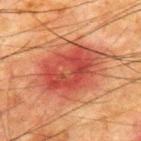Impression: No biopsy was performed on this lesion — it was imaged during a full skin examination and was not determined to be concerning. Acquisition and patient details: A roughly 15 mm field-of-view crop from a total-body skin photograph. A male patient, aged around 65. Imaged with cross-polarized lighting. The recorded lesion diameter is about 11 mm. An algorithmic analysis of the crop reported a lesion area of about 46 mm², an eccentricity of roughly 0.65, and two-axis asymmetry of about 0.2. The software also gave internal color variation of about 7 on a 0–10 scale and radial color variation of about 2.5. The analysis additionally found a classifier nevus-likeness of about 5/100 and a detector confidence of about 100 out of 100 that the crop contains a lesion. The lesion is located on the upper back.